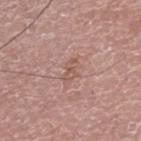This lesion was catalogued during total-body skin photography and was not selected for biopsy.